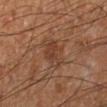<case>
<biopsy_status>not biopsied; imaged during a skin examination</biopsy_status>
<image>
  <source>total-body photography crop</source>
  <field_of_view_mm>15</field_of_view_mm>
</image>
<patient>
  <sex>male</sex>
  <age_approx>60</age_approx>
</patient>
<lesion_size>
  <long_diameter_mm_approx>4.5</long_diameter_mm_approx>
</lesion_size>
<site>left lower leg</site>
<lighting>cross-polarized</lighting>
</case>